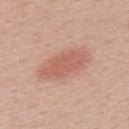No biopsy was performed on this lesion — it was imaged during a full skin examination and was not determined to be concerning. The subject is a female approximately 40 years of age. The lesion is on the upper back. A region of skin cropped from a whole-body photographic capture, roughly 15 mm wide. The total-body-photography lesion software estimated a footprint of about 16 mm² and a shape-asymmetry score of about 0.15 (0 = symmetric). The analysis additionally found a lesion color around L≈60 a*≈24 b*≈29 in CIELAB, a lesion–skin lightness drop of about 9, and a normalized lesion–skin contrast near 6. And it measured a nevus-likeness score of about 100/100 and a lesion-detection confidence of about 100/100. Measured at roughly 6 mm in maximum diameter.